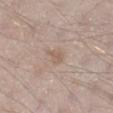Imaged during a routine full-body skin examination; the lesion was not biopsied and no histopathology is available.